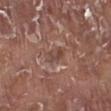The lesion was photographed on a routine skin check and not biopsied; there is no pathology result.
Located on the right lower leg.
A male subject, aged around 75.
About 2.5 mm across.
Automated image analysis of the tile measured an automated nevus-likeness rating near 0 out of 100 and lesion-presence confidence of about 50/100.
A 15 mm close-up tile from a total-body photography series done for melanoma screening.